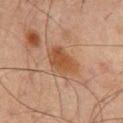biopsy status — total-body-photography surveillance lesion; no biopsy | patient — male, in their mid-60s | diameter — ≈4 mm | location — the upper back | acquisition — 15 mm crop, total-body photography | illumination — cross-polarized | automated metrics — a footprint of about 10 mm² and a shape-asymmetry score of about 0.25 (0 = symmetric); an average lesion color of about L≈40 a*≈18 b*≈29 (CIELAB) and a lesion-to-skin contrast of about 7.5 (normalized; higher = more distinct); border irregularity of about 3 on a 0–10 scale and a color-variation rating of about 3/10.